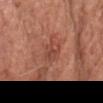follow-up: catalogued during a skin exam; not biopsied | subject: male, in their 80s | body site: the head or neck | image: 15 mm crop, total-body photography.